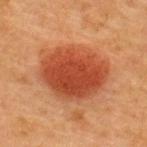No biopsy was performed on this lesion — it was imaged during a full skin examination and was not determined to be concerning.
Captured under cross-polarized illumination.
Measured at roughly 7.5 mm in maximum diameter.
This image is a 15 mm lesion crop taken from a total-body photograph.
The subject is a female aged 38–42.
On the back.
Automated tile analysis of the lesion measured a shape-asymmetry score of about 0.1 (0 = symmetric). And it measured a mean CIELAB color near L≈42 a*≈29 b*≈34, roughly 12 lightness units darker than nearby skin, and a normalized lesion–skin contrast near 9.5.